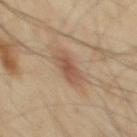Findings:
- workup · no biopsy performed (imaged during a skin exam)
- image source · ~15 mm tile from a whole-body skin photo
- anatomic site · the mid back
- subject · male, approximately 55 years of age